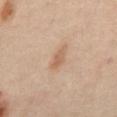- notes — imaged on a skin check; not biopsied
- subject — male, approximately 65 years of age
- lesion diameter — about 3 mm
- image source — ~15 mm tile from a whole-body skin photo
- tile lighting — cross-polarized illumination
- anatomic site — the mid back
- image-analysis metrics — a lesion area of about 4 mm², an outline eccentricity of about 0.85 (0 = round, 1 = elongated), and a symmetry-axis asymmetry near 0.3; a classifier nevus-likeness of about 55/100 and a lesion-detection confidence of about 100/100